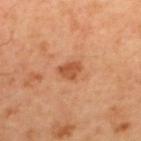Imaged during a routine full-body skin examination; the lesion was not biopsied and no histopathology is available. From the back. The lesion's longest dimension is about 2.5 mm. Captured under cross-polarized illumination. A close-up tile cropped from a whole-body skin photograph, about 15 mm across. The patient is a male roughly 60 years of age. Automated image analysis of the tile measured a footprint of about 4.5 mm², a shape eccentricity near 0.65, and a shape-asymmetry score of about 0.3 (0 = symmetric). The analysis additionally found an average lesion color of about L≈51 a*≈27 b*≈38 (CIELAB), about 10 CIELAB-L* units darker than the surrounding skin, and a normalized border contrast of about 7.5. And it measured an automated nevus-likeness rating near 60 out of 100 and lesion-presence confidence of about 100/100.